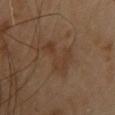  biopsy_status: not biopsied; imaged during a skin examination
  lighting: cross-polarized
  site: upper back
  patient:
    sex: female
    age_approx: 40
  image:
    source: total-body photography crop
    field_of_view_mm: 15